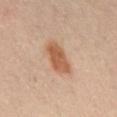lighting: cross-polarized illumination | subject: male, roughly 65 years of age | TBP lesion metrics: an area of roughly 9 mm², an eccentricity of roughly 0.8, and a symmetry-axis asymmetry near 0.2; a lesion color around L≈57 a*≈21 b*≈34 in CIELAB, roughly 12 lightness units darker than nearby skin, and a lesion-to-skin contrast of about 8.5 (normalized; higher = more distinct); a border-irregularity index near 2.5/10, a color-variation rating of about 3.5/10, and radial color variation of about 1 | imaging modality: 15 mm crop, total-body photography | body site: the mid back.